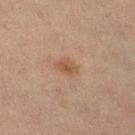Q: Was a biopsy performed?
A: total-body-photography surveillance lesion; no biopsy
Q: What are the patient's age and sex?
A: female, aged 18–22
Q: What is the imaging modality?
A: 15 mm crop, total-body photography
Q: Where on the body is the lesion?
A: the right lower leg
Q: Lesion size?
A: ~3 mm (longest diameter)
Q: What lighting was used for the tile?
A: cross-polarized